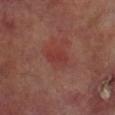biopsy status: no biopsy performed (imaged during a skin exam); body site: the right lower leg; image: ~15 mm crop, total-body skin-cancer survey; patient: male, aged 68 to 72; lighting: cross-polarized; diameter: about 2.5 mm.